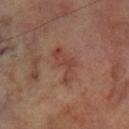Recorded during total-body skin imaging; not selected for excision or biopsy. The patient is a male approximately 70 years of age. Approximately 4.5 mm at its widest. A region of skin cropped from a whole-body photographic capture, roughly 15 mm wide. On the left lower leg. Captured under cross-polarized illumination.